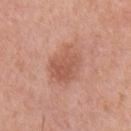| field | value |
|---|---|
| follow-up | imaged on a skin check; not biopsied |
| site | the chest |
| imaging modality | 15 mm crop, total-body photography |
| subject | male, aged 68 to 72 |
| lesion size | ≈4.5 mm |
| automated metrics | a shape eccentricity near 0.65; a mean CIELAB color near L≈56 a*≈25 b*≈30, about 9 CIELAB-L* units darker than the surrounding skin, and a normalized border contrast of about 6; border irregularity of about 2 on a 0–10 scale, a color-variation rating of about 3/10, and radial color variation of about 1; a nevus-likeness score of about 25/100 and a lesion-detection confidence of about 100/100 |